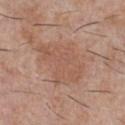notes — no biopsy performed (imaged during a skin exam)
size — ~6.5 mm (longest diameter)
illumination — white-light
image source — ~15 mm tile from a whole-body skin photo
patient — male, about 30 years old
location — the chest